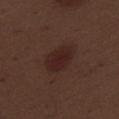Q: Was a biopsy performed?
A: catalogued during a skin exam; not biopsied
Q: Automated lesion metrics?
A: an area of roughly 9.5 mm² and two-axis asymmetry of about 0.15; roughly 6 lightness units darker than nearby skin and a normalized lesion–skin contrast near 7.5; border irregularity of about 1.5 on a 0–10 scale, internal color variation of about 2 on a 0–10 scale, and peripheral color asymmetry of about 0.5; an automated nevus-likeness rating near 80 out of 100 and a detector confidence of about 100 out of 100 that the crop contains a lesion
Q: Who is the patient?
A: male, roughly 70 years of age
Q: What lighting was used for the tile?
A: white-light illumination
Q: Lesion size?
A: ~4.5 mm (longest diameter)
Q: What kind of image is this?
A: 15 mm crop, total-body photography
Q: What is the anatomic site?
A: the right thigh